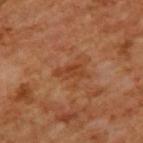Clinical impression: This lesion was catalogued during total-body skin photography and was not selected for biopsy. Clinical summary: The lesion is located on the back. Cropped from a whole-body photographic skin survey; the tile spans about 15 mm. Automated tile analysis of the lesion measured a nevus-likeness score of about 0/100 and a lesion-detection confidence of about 100/100. The tile uses cross-polarized illumination. A male subject aged approximately 65. Measured at roughly 3.5 mm in maximum diameter.